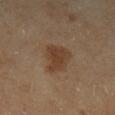Impression: This lesion was catalogued during total-body skin photography and was not selected for biopsy. Acquisition and patient details: The tile uses cross-polarized illumination. The subject is a female aged 58–62. On the left lower leg. Measured at roughly 4 mm in maximum diameter. Cropped from a whole-body photographic skin survey; the tile spans about 15 mm.